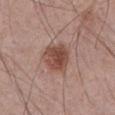Impression:
Part of a total-body skin-imaging series; this lesion was reviewed on a skin check and was not flagged for biopsy.
Context:
Automated tile analysis of the lesion measured a lesion area of about 10 mm², an outline eccentricity of about 0.55 (0 = round, 1 = elongated), and a shape-asymmetry score of about 0.25 (0 = symmetric). The analysis additionally found an average lesion color of about L≈47 a*≈22 b*≈25 (CIELAB) and a lesion–skin lightness drop of about 12. The subject is a male in their 70s. Imaged with white-light lighting. About 4 mm across. A region of skin cropped from a whole-body photographic capture, roughly 15 mm wide. The lesion is located on the abdomen.